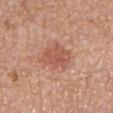{
  "lesion_size": {
    "long_diameter_mm_approx": 4.0
  },
  "patient": {
    "sex": "female",
    "age_approx": 50
  },
  "lighting": "white-light",
  "site": "arm",
  "image": {
    "source": "total-body photography crop",
    "field_of_view_mm": 15
  }
}